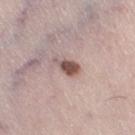automated lesion analysis: an outline eccentricity of about 0.8 (0 = round, 1 = elongated); a lesion–skin lightness drop of about 15 and a lesion-to-skin contrast of about 10 (normalized; higher = more distinct)
acquisition: total-body-photography crop, ~15 mm field of view
lesion size: ~3 mm (longest diameter)
illumination: white-light illumination
subject: male, in their mid-50s
location: the right thigh